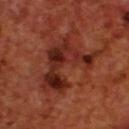Impression:
Captured during whole-body skin photography for melanoma surveillance; the lesion was not biopsied.
Image and clinical context:
About 8.5 mm across. Cropped from a total-body skin-imaging series; the visible field is about 15 mm. From the upper back. The patient is a male aged around 70.